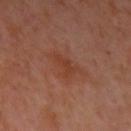– follow-up: imaged on a skin check; not biopsied
– imaging modality: ~15 mm crop, total-body skin-cancer survey
– lesion diameter: about 3 mm
– illumination: cross-polarized illumination
– site: the arm
– patient: female, approximately 50 years of age